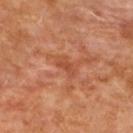Assessment:
This lesion was catalogued during total-body skin photography and was not selected for biopsy.
Context:
The patient is a female approximately 50 years of age. Cropped from a total-body skin-imaging series; the visible field is about 15 mm. This is a cross-polarized tile. From the back.